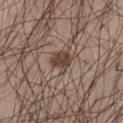Case summary:
– lighting · white-light illumination
– size · ≈3 mm
– patient · male, aged around 55
– image source · ~15 mm tile from a whole-body skin photo
– site · the left thigh